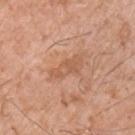No biopsy was performed on this lesion — it was imaged during a full skin examination and was not determined to be concerning.
From the chest.
This image is a 15 mm lesion crop taken from a total-body photograph.
A male subject roughly 75 years of age.
The tile uses white-light illumination.